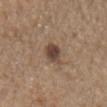notes: no biopsy performed (imaged during a skin exam); patient: male, aged 68–72; site: the chest; image source: ~15 mm crop, total-body skin-cancer survey.